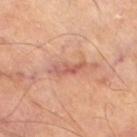The lesion was photographed on a routine skin check and not biopsied; there is no pathology result. The lesion is on the right thigh. A 15 mm crop from a total-body photograph taken for skin-cancer surveillance. A male subject, about 70 years old. Longest diameter approximately 4 mm. Captured under cross-polarized illumination.